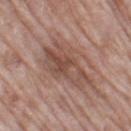No biopsy was performed on this lesion — it was imaged during a full skin examination and was not determined to be concerning. Imaged with white-light lighting. A female patient roughly 75 years of age. Located on the left thigh. Cropped from a whole-body photographic skin survey; the tile spans about 15 mm. The lesion-visualizer software estimated a lesion color around L≈49 a*≈19 b*≈25 in CIELAB and a normalized lesion–skin contrast near 6.5. Approximately 6.5 mm at its widest.